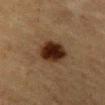Image and clinical context: A female patient aged around 55. A 15 mm close-up extracted from a 3D total-body photography capture. An algorithmic analysis of the crop reported an average lesion color of about L≈23 a*≈15 b*≈23 (CIELAB), a lesion–skin lightness drop of about 13, and a lesion-to-skin contrast of about 14 (normalized; higher = more distinct). The software also gave a classifier nevus-likeness of about 100/100 and a lesion-detection confidence of about 100/100. Located on the abdomen. About 4.5 mm across.